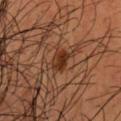Assessment: The lesion was tiled from a total-body skin photograph and was not biopsied. Image and clinical context: The subject is a male in their mid- to late 30s. Imaged with cross-polarized lighting. A region of skin cropped from a whole-body photographic capture, roughly 15 mm wide. On the head or neck. An algorithmic analysis of the crop reported an eccentricity of roughly 0.65 and a shape-asymmetry score of about 0.25 (0 = symmetric). And it measured a border-irregularity index near 2.5/10 and radial color variation of about 1.5.